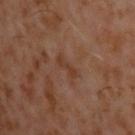Q: Is there a histopathology result?
A: no biopsy performed (imaged during a skin exam)
Q: Where on the body is the lesion?
A: the upper back
Q: What is the lesion's diameter?
A: about 3 mm
Q: Who is the patient?
A: male, approximately 60 years of age
Q: What is the imaging modality?
A: total-body-photography crop, ~15 mm field of view
Q: Illumination type?
A: cross-polarized